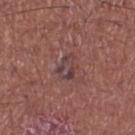Recorded during total-body skin imaging; not selected for excision or biopsy. Located on the left thigh. A male patient, aged around 65. A lesion tile, about 15 mm wide, cut from a 3D total-body photograph.